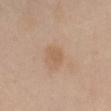Assessment: Captured during whole-body skin photography for melanoma surveillance; the lesion was not biopsied. Clinical summary: Automated tile analysis of the lesion measured a shape eccentricity near 0.7 and a shape-asymmetry score of about 0.2 (0 = symmetric). The software also gave an average lesion color of about L≈59 a*≈17 b*≈32 (CIELAB) and a lesion-to-skin contrast of about 5 (normalized; higher = more distinct). The software also gave internal color variation of about 1 on a 0–10 scale and radial color variation of about 0.5. It also reported a nevus-likeness score of about 0/100. Measured at roughly 2.5 mm in maximum diameter. A female subject, roughly 55 years of age. Cropped from a whole-body photographic skin survey; the tile spans about 15 mm. On the back. The tile uses cross-polarized illumination.